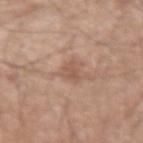{
  "biopsy_status": "not biopsied; imaged during a skin examination",
  "patient": {
    "sex": "male",
    "age_approx": 60
  },
  "image": {
    "source": "total-body photography crop",
    "field_of_view_mm": 15
  },
  "site": "right upper arm",
  "automated_metrics": {
    "area_mm2_approx": 3.0,
    "eccentricity": 0.85,
    "shape_asymmetry": 0.4,
    "cielab_L": 54,
    "cielab_a": 20,
    "cielab_b": 28,
    "vs_skin_darker_L": 8.0,
    "vs_skin_contrast_norm": 5.5
  },
  "lighting": "white-light",
  "lesion_size": {
    "long_diameter_mm_approx": 2.5
  }
}